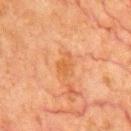notes: no biopsy performed (imaged during a skin exam)
automated metrics: a lesion area of about 4 mm², an eccentricity of roughly 0.7, and a symmetry-axis asymmetry near 0.4; about 6 CIELAB-L* units darker than the surrounding skin
lesion diameter: about 3 mm
location: the chest
acquisition: ~15 mm tile from a whole-body skin photo
subject: male, aged 78–82
lighting: cross-polarized illumination